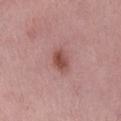A region of skin cropped from a whole-body photographic capture, roughly 15 mm wide. The subject is a female aged 48–52. On the right lower leg.